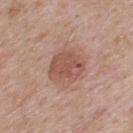– biopsy status: no biopsy performed (imaged during a skin exam)
– image: 15 mm crop, total-body photography
– tile lighting: white-light illumination
– patient: male, aged approximately 50
– anatomic site: the upper back
– size: ≈4 mm